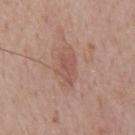Notes:
- notes: catalogued during a skin exam; not biopsied